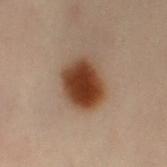{"biopsy_status": "not biopsied; imaged during a skin examination", "image": {"source": "total-body photography crop", "field_of_view_mm": 15}, "patient": {"sex": "female", "age_approx": 60}, "site": "right leg", "lesion_size": {"long_diameter_mm_approx": 4.5}, "lighting": "cross-polarized"}